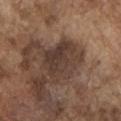The lesion was tiled from a total-body skin photograph and was not biopsied. This image is a 15 mm lesion crop taken from a total-body photograph. A male subject, roughly 75 years of age. This is a white-light tile. Located on the arm.